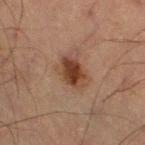follow-up: imaged on a skin check; not biopsied | site: the left thigh | tile lighting: cross-polarized | automated metrics: an outline eccentricity of about 0.8 (0 = round, 1 = elongated) and a shape-asymmetry score of about 0.2 (0 = symmetric); a border-irregularity index near 2/10 and a within-lesion color-variation index near 3/10; a classifier nevus-likeness of about 95/100 | imaging modality: ~15 mm tile from a whole-body skin photo | subject: male, approximately 65 years of age.